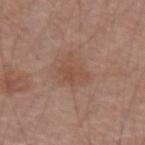Q: Is there a histopathology result?
A: imaged on a skin check; not biopsied
Q: What are the patient's age and sex?
A: male, aged 58 to 62
Q: How large is the lesion?
A: ≈3.5 mm
Q: What did automated image analysis measure?
A: an area of roughly 6.5 mm², a shape eccentricity near 0.7, and two-axis asymmetry of about 0.35; a mean CIELAB color near L≈49 a*≈20 b*≈28, about 6 CIELAB-L* units darker than the surrounding skin, and a normalized border contrast of about 5.5
Q: How was this image acquired?
A: 15 mm crop, total-body photography
Q: Illumination type?
A: white-light illumination
Q: Lesion location?
A: the right forearm